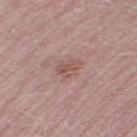Impression: Imaged during a routine full-body skin examination; the lesion was not biopsied and no histopathology is available. Clinical summary: From the right thigh. Imaged with white-light lighting. Automated tile analysis of the lesion measured an area of roughly 4.5 mm² and an outline eccentricity of about 0.8 (0 = round, 1 = elongated). It also reported an average lesion color of about L≈54 a*≈20 b*≈23 (CIELAB) and about 8 CIELAB-L* units darker than the surrounding skin. A male patient about 50 years old. A lesion tile, about 15 mm wide, cut from a 3D total-body photograph.